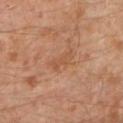Recorded during total-body skin imaging; not selected for excision or biopsy. Imaged with cross-polarized lighting. The subject is a male approximately 30 years of age. An algorithmic analysis of the crop reported a lesion color around L≈51 a*≈22 b*≈34 in CIELAB and a normalized lesion–skin contrast near 5. It also reported a classifier nevus-likeness of about 0/100. Located on the left lower leg. A close-up tile cropped from a whole-body skin photograph, about 15 mm across.